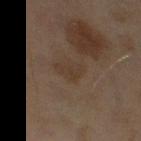The lesion was photographed on a routine skin check and not biopsied; there is no pathology result. Automated image analysis of the tile measured a mean CIELAB color near L≈32 a*≈12 b*≈22, roughly 5 lightness units darker than nearby skin, and a normalized border contrast of about 5. Cropped from a whole-body photographic skin survey; the tile spans about 15 mm. Longest diameter approximately 3 mm. On the left thigh. The patient is a female about 60 years old.